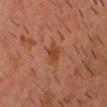  biopsy_status: not biopsied; imaged during a skin examination
  image:
    source: total-body photography crop
    field_of_view_mm: 15
  patient:
    sex: male
    age_approx: 50
  site: head or neck